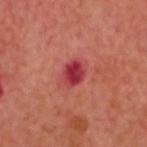notes = total-body-photography surveillance lesion; no biopsy | anatomic site = the head or neck | TBP lesion metrics = border irregularity of about 1.5 on a 0–10 scale, a within-lesion color-variation index near 4/10, and a peripheral color-asymmetry measure near 1.5; a lesion-detection confidence of about 100/100 | subject = male, in their 50s | imaging modality = ~15 mm tile from a whole-body skin photo.